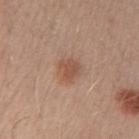Assessment:
The lesion was photographed on a routine skin check and not biopsied; there is no pathology result.
Context:
Imaged with white-light lighting. The lesion is located on the right upper arm. A male patient, aged 28 to 32. A 15 mm close-up tile from a total-body photography series done for melanoma screening. The lesion-visualizer software estimated a lesion area of about 5.5 mm² and a shape-asymmetry score of about 0.25 (0 = symmetric). And it measured a detector confidence of about 100 out of 100 that the crop contains a lesion.